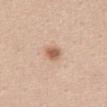Assessment:
No biopsy was performed on this lesion — it was imaged during a full skin examination and was not determined to be concerning.
Clinical summary:
On the abdomen. Automated image analysis of the tile measured a lesion–skin lightness drop of about 13 and a lesion-to-skin contrast of about 8.5 (normalized; higher = more distinct). And it measured border irregularity of about 2 on a 0–10 scale, a within-lesion color-variation index near 3.5/10, and radial color variation of about 1. And it measured a detector confidence of about 100 out of 100 that the crop contains a lesion. Cropped from a total-body skin-imaging series; the visible field is about 15 mm. The tile uses white-light illumination. A female subject, aged 43–47.